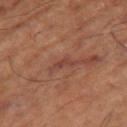  biopsy_status: not biopsied; imaged during a skin examination